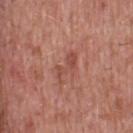workup — total-body-photography surveillance lesion; no biopsy
automated metrics — a footprint of about 4.5 mm², an eccentricity of roughly 0.9, and two-axis asymmetry of about 0.5; a mean CIELAB color near L≈49 a*≈26 b*≈28, roughly 8 lightness units darker than nearby skin, and a lesion-to-skin contrast of about 5.5 (normalized; higher = more distinct); a color-variation rating of about 1/10 and a peripheral color-asymmetry measure near 0.5
image source — ~15 mm tile from a whole-body skin photo
lesion diameter — ~3.5 mm (longest diameter)
patient — male, aged 48–52
location — the upper back
lighting — white-light illumination